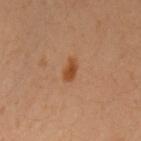Q: Is there a histopathology result?
A: imaged on a skin check; not biopsied
Q: What are the patient's age and sex?
A: female, about 40 years old
Q: How was this image acquired?
A: 15 mm crop, total-body photography
Q: What is the anatomic site?
A: the left upper arm
Q: How was the tile lit?
A: cross-polarized
Q: How large is the lesion?
A: ≈2.5 mm